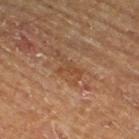Q: Was a biopsy performed?
A: total-body-photography surveillance lesion; no biopsy
Q: Lesion size?
A: ~3 mm (longest diameter)
Q: What is the imaging modality?
A: 15 mm crop, total-body photography
Q: Illumination type?
A: cross-polarized illumination
Q: What is the anatomic site?
A: the right thigh
Q: Who is the patient?
A: male, approximately 65 years of age